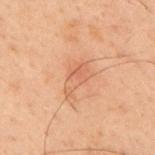The lesion-visualizer software estimated a footprint of about 6.5 mm², a shape eccentricity near 0.8, and a symmetry-axis asymmetry near 0.4. The analysis additionally found a lesion–skin lightness drop of about 6 and a normalized border contrast of about 4.5. The software also gave a border-irregularity rating of about 4/10. It also reported a classifier nevus-likeness of about 0/100 and lesion-presence confidence of about 100/100. Cropped from a total-body skin-imaging series; the visible field is about 15 mm. Measured at roughly 3.5 mm in maximum diameter. A male subject, roughly 60 years of age. Located on the upper back.